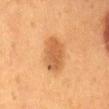biopsy status: total-body-photography surveillance lesion; no biopsy | patient: female, aged approximately 50 | image: ~15 mm crop, total-body skin-cancer survey | body site: the back.